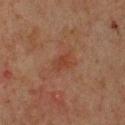This lesion was catalogued during total-body skin photography and was not selected for biopsy. The lesion-visualizer software estimated a mean CIELAB color near L≈33 a*≈21 b*≈26, about 5 CIELAB-L* units darker than the surrounding skin, and a normalized border contrast of about 5.5. This is a cross-polarized tile. The lesion's longest dimension is about 2.5 mm. Cropped from a total-body skin-imaging series; the visible field is about 15 mm. The lesion is located on the front of the torso. A male subject, aged 43 to 47.